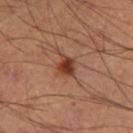Case summary:
– size: about 2.5 mm
– automated metrics: a lesion area of about 4.5 mm² and an eccentricity of roughly 0.5; a nevus-likeness score of about 90/100 and a detector confidence of about 100 out of 100 that the crop contains a lesion
– body site: the right thigh
– subject: male, about 60 years old
– imaging modality: total-body-photography crop, ~15 mm field of view
– illumination: cross-polarized